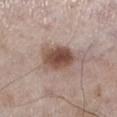| feature | finding |
|---|---|
| patient | male, in their 60s |
| illumination | white-light |
| image source | ~15 mm tile from a whole-body skin photo |
| anatomic site | the left lower leg |